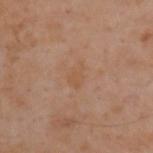No biopsy was performed on this lesion — it was imaged during a full skin examination and was not determined to be concerning. Cropped from a total-body skin-imaging series; the visible field is about 15 mm. Located on the upper back. The subject is a male aged 53 to 57.